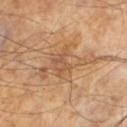– notes — total-body-photography surveillance lesion; no biopsy
– illumination — cross-polarized
– size — ≈7 mm
– image source — 15 mm crop, total-body photography
– subject — male, in their mid- to late 60s